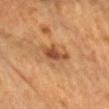Recorded during total-body skin imaging; not selected for excision or biopsy. A female subject, about 65 years old. This image is a 15 mm lesion crop taken from a total-body photograph. The lesion is located on the head or neck. About 3.5 mm across. This is a cross-polarized tile.